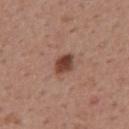Assessment:
This lesion was catalogued during total-body skin photography and was not selected for biopsy.
Image and clinical context:
The lesion is located on the mid back. A roughly 15 mm field-of-view crop from a total-body skin photograph. Automated image analysis of the tile measured an eccentricity of roughly 0.7. The software also gave internal color variation of about 3.5 on a 0–10 scale and peripheral color asymmetry of about 1. The lesion's longest dimension is about 2.5 mm. A male patient, approximately 55 years of age.